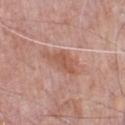Recorded during total-body skin imaging; not selected for excision or biopsy. This is a white-light tile. The lesion is on the chest. The subject is a male roughly 70 years of age. Cropped from a whole-body photographic skin survey; the tile spans about 15 mm. About 4.5 mm across.